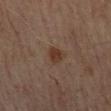Impression: Imaged during a routine full-body skin examination; the lesion was not biopsied and no histopathology is available. Acquisition and patient details: This is a cross-polarized tile. The lesion is on the chest. About 3 mm across. Cropped from a whole-body photographic skin survey; the tile spans about 15 mm. A male patient, aged around 70.